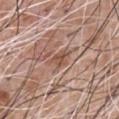Clinical impression: No biopsy was performed on this lesion — it was imaged during a full skin examination and was not determined to be concerning. Background: A male patient aged 58–62. The lesion is located on the front of the torso. Cropped from a total-body skin-imaging series; the visible field is about 15 mm. Longest diameter approximately 3 mm.